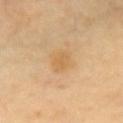notes — imaged on a skin check; not biopsied | patient — female, approximately 60 years of age | body site — the chest | image-analysis metrics — an average lesion color of about L≈64 a*≈18 b*≈43 (CIELAB), about 6 CIELAB-L* units darker than the surrounding skin, and a lesion-to-skin contrast of about 5.5 (normalized; higher = more distinct); border irregularity of about 2 on a 0–10 scale, internal color variation of about 1.5 on a 0–10 scale, and a peripheral color-asymmetry measure near 0.5; an automated nevus-likeness rating near 0 out of 100 and a lesion-detection confidence of about 100/100 | tile lighting — cross-polarized | lesion size — ≈2.5 mm | image source — 15 mm crop, total-body photography.